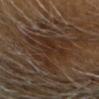{
  "automated_metrics": {
    "area_mm2_approx": 21.0,
    "eccentricity": 0.4,
    "shape_asymmetry": 0.45,
    "lesion_detection_confidence_0_100": 70
  },
  "image": {
    "source": "total-body photography crop",
    "field_of_view_mm": 15
  },
  "site": "head or neck",
  "patient": {
    "sex": "male",
    "age_approx": 65
  },
  "lighting": "cross-polarized",
  "lesion_size": {
    "long_diameter_mm_approx": 6.5
  }
}